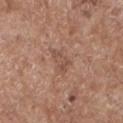The lesion was photographed on a routine skin check and not biopsied; there is no pathology result.
From the left upper arm.
The lesion-visualizer software estimated an area of roughly 4.5 mm², a shape eccentricity near 0.85, and a symmetry-axis asymmetry near 0.4. It also reported a lesion color around L≈50 a*≈20 b*≈28 in CIELAB, a lesion–skin lightness drop of about 7, and a normalized border contrast of about 5. It also reported a border-irregularity rating of about 4/10, a within-lesion color-variation index near 2.5/10, and a peripheral color-asymmetry measure near 1. And it measured a lesion-detection confidence of about 100/100.
Cropped from a total-body skin-imaging series; the visible field is about 15 mm.
About 3 mm across.
A male subject, aged approximately 70.